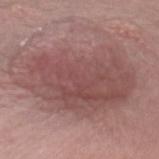This lesion was catalogued during total-body skin photography and was not selected for biopsy. Imaged with white-light lighting. The subject is a female in their mid- to late 60s. Longest diameter approximately 12 mm. The lesion-visualizer software estimated a lesion area of about 60 mm² and two-axis asymmetry of about 0.25. It also reported a mean CIELAB color near L≈49 a*≈22 b*≈20, roughly 10 lightness units darker than nearby skin, and a normalized border contrast of about 7.5. On the chest. A 15 mm crop from a total-body photograph taken for skin-cancer surveillance.